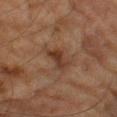| feature | finding |
|---|---|
| lighting | cross-polarized illumination |
| diameter | ≈3.5 mm |
| automated metrics | border irregularity of about 4 on a 0–10 scale; an automated nevus-likeness rating near 0 out of 100 and a detector confidence of about 100 out of 100 that the crop contains a lesion |
| image | total-body-photography crop, ~15 mm field of view |
| body site | the left thigh |
| subject | male, about 85 years old or older |